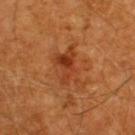The lesion was tiled from a total-body skin photograph and was not biopsied.
A lesion tile, about 15 mm wide, cut from a 3D total-body photograph.
About 5 mm across.
A male patient, aged approximately 60.
From the upper back.